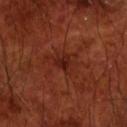Clinical impression:
The lesion was photographed on a routine skin check and not biopsied; there is no pathology result.
Context:
A roughly 15 mm field-of-view crop from a total-body skin photograph. Located on the front of the torso. Automated tile analysis of the lesion measured an automated nevus-likeness rating near 0 out of 100. The tile uses cross-polarized illumination. A male subject, about 70 years old.